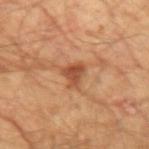Imaged during a routine full-body skin examination; the lesion was not biopsied and no histopathology is available. Longest diameter approximately 3 mm. This is a cross-polarized tile. A 15 mm close-up extracted from a 3D total-body photography capture. From the left upper arm. The subject is a male aged 63–67.